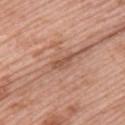{
  "biopsy_status": "not biopsied; imaged during a skin examination",
  "image": {
    "source": "total-body photography crop",
    "field_of_view_mm": 15
  },
  "patient": {
    "sex": "female",
    "age_approx": 60
  },
  "site": "upper back",
  "automated_metrics": {
    "eccentricity": 0.9,
    "shape_asymmetry": 0.35,
    "cielab_L": 53,
    "cielab_a": 23,
    "cielab_b": 30,
    "vs_skin_darker_L": 9.0,
    "vs_skin_contrast_norm": 6.5,
    "lesion_detection_confidence_0_100": 65
  },
  "lesion_size": {
    "long_diameter_mm_approx": 3.0
  },
  "lighting": "white-light"
}